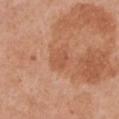Notes:
- workup: total-body-photography surveillance lesion; no biopsy
- site: the front of the torso
- image source: ~15 mm crop, total-body skin-cancer survey
- illumination: white-light
- size: ~2.5 mm (longest diameter)
- subject: female, aged 53 to 57